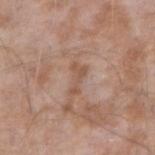Part of a total-body skin-imaging series; this lesion was reviewed on a skin check and was not flagged for biopsy.
A male subject roughly 75 years of age.
A 15 mm close-up extracted from a 3D total-body photography capture.
The lesion is located on the right forearm.